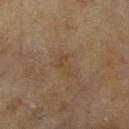The lesion was tiled from a total-body skin photograph and was not biopsied. A male subject aged around 65. A close-up tile cropped from a whole-body skin photograph, about 15 mm across. The lesion is located on the right lower leg.